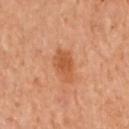Recorded during total-body skin imaging; not selected for excision or biopsy.
An algorithmic analysis of the crop reported a border-irregularity rating of about 2.5/10 and internal color variation of about 2 on a 0–10 scale. And it measured an automated nevus-likeness rating near 40 out of 100 and a detector confidence of about 100 out of 100 that the crop contains a lesion.
A female patient aged approximately 60.
About 3.5 mm across.
From the arm.
A close-up tile cropped from a whole-body skin photograph, about 15 mm across.
The tile uses cross-polarized illumination.